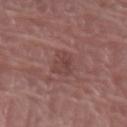<case>
<biopsy_status>not biopsied; imaged during a skin examination</biopsy_status>
<lighting>white-light</lighting>
<automated_metrics>
  <cielab_L>43</cielab_L>
  <cielab_a>22</cielab_a>
  <cielab_b>21</cielab_b>
  <vs_skin_darker_L>6.0</vs_skin_darker_L>
  <vs_skin_contrast_norm>5.0</vs_skin_contrast_norm>
  <nevus_likeness_0_100>0</nevus_likeness_0_100>
</automated_metrics>
<patient>
  <sex>female</sex>
  <age_approx>70</age_approx>
</patient>
<site>arm</site>
<image>
  <source>total-body photography crop</source>
  <field_of_view_mm>15</field_of_view_mm>
</image>
<lesion_size>
  <long_diameter_mm_approx>3.5</long_diameter_mm_approx>
</lesion_size>
</case>